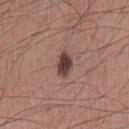The lesion was photographed on a routine skin check and not biopsied; there is no pathology result.
The lesion-visualizer software estimated two-axis asymmetry of about 0.2. The software also gave a lesion color around L≈40 a*≈19 b*≈21 in CIELAB, roughly 16 lightness units darker than nearby skin, and a lesion-to-skin contrast of about 12 (normalized; higher = more distinct). The software also gave a border-irregularity index near 2/10, a within-lesion color-variation index near 3/10, and radial color variation of about 1.
The lesion is on the mid back.
A 15 mm crop from a total-body photograph taken for skin-cancer surveillance.
A male patient aged 33 to 37.
The tile uses white-light illumination.